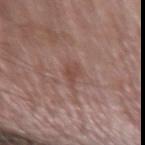The lesion was tiled from a total-body skin photograph and was not biopsied. Imaged with white-light lighting. A male subject in their mid- to late 70s. A lesion tile, about 15 mm wide, cut from a 3D total-body photograph. From the left forearm. About 3 mm across. The lesion-visualizer software estimated a border-irregularity index near 5/10 and a peripheral color-asymmetry measure near 0.5. And it measured an automated nevus-likeness rating near 0 out of 100 and a lesion-detection confidence of about 100/100.